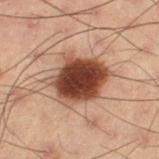<case>
  <biopsy_status>not biopsied; imaged during a skin examination</biopsy_status>
  <site>left thigh</site>
  <patient>
    <sex>male</sex>
    <age_approx>55</age_approx>
  </patient>
  <lesion_size>
    <long_diameter_mm_approx>6.0</long_diameter_mm_approx>
  </lesion_size>
  <image>
    <source>total-body photography crop</source>
    <field_of_view_mm>15</field_of_view_mm>
  </image>
</case>